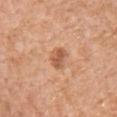location = the upper back; subject = male, approximately 60 years of age; imaging modality = ~15 mm tile from a whole-body skin photo; size = about 3 mm; tile lighting = white-light.